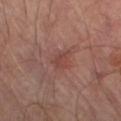notes — no biopsy performed (imaged during a skin exam) | lesion size — ≈2.5 mm | subject — male, roughly 70 years of age | image source — 15 mm crop, total-body photography | anatomic site — the left thigh | illumination — cross-polarized illumination.